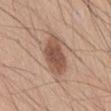The lesion is on the abdomen. The recorded lesion diameter is about 5.5 mm. A region of skin cropped from a whole-body photographic capture, roughly 15 mm wide. The tile uses white-light illumination. A male subject aged 58 to 62.